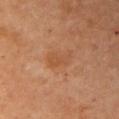Case summary:
- workup: imaged on a skin check; not biopsied
- location: the right upper arm
- patient: female, aged 68 to 72
- image source: ~15 mm crop, total-body skin-cancer survey